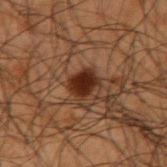A male patient, aged approximately 50. The tile uses cross-polarized illumination. On the right upper arm. Automated tile analysis of the lesion measured a border-irregularity rating of about 2/10, a color-variation rating of about 3.5/10, and radial color variation of about 1. Measured at roughly 3 mm in maximum diameter. Cropped from a total-body skin-imaging series; the visible field is about 15 mm.